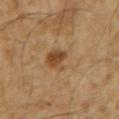Captured during whole-body skin photography for melanoma surveillance; the lesion was not biopsied. A male patient, aged approximately 60. The tile uses cross-polarized illumination. Approximately 5 mm at its widest. The lesion is on the right upper arm. A close-up tile cropped from a whole-body skin photograph, about 15 mm across.